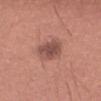Captured during whole-body skin photography for melanoma surveillance; the lesion was not biopsied. A male patient, about 65 years old. Located on the lower back. A close-up tile cropped from a whole-body skin photograph, about 15 mm across.